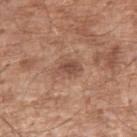Recorded during total-body skin imaging; not selected for excision or biopsy. A male subject aged approximately 65. About 3 mm across. Captured under white-light illumination. A roughly 15 mm field-of-view crop from a total-body skin photograph. Automated image analysis of the tile measured a footprint of about 5 mm², an eccentricity of roughly 0.75, and a shape-asymmetry score of about 0.3 (0 = symmetric). It also reported a border-irregularity index near 3/10, a color-variation rating of about 2/10, and a peripheral color-asymmetry measure near 1. On the left upper arm.